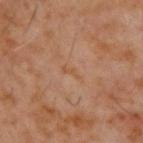notes: imaged on a skin check; not biopsied
TBP lesion metrics: internal color variation of about 0 on a 0–10 scale and radial color variation of about 0
subject: male, roughly 60 years of age
image source: total-body-photography crop, ~15 mm field of view
tile lighting: cross-polarized illumination
diameter: about 2.5 mm
anatomic site: the upper back